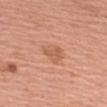Assessment:
Recorded during total-body skin imaging; not selected for excision or biopsy.
Background:
Longest diameter approximately 3 mm. Located on the left upper arm. This is a white-light tile. Cropped from a total-body skin-imaging series; the visible field is about 15 mm. The subject is a male aged 58–62.